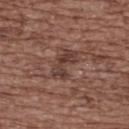notes — imaged on a skin check; not biopsied
size — ~4 mm (longest diameter)
TBP lesion metrics — a footprint of about 6 mm² and two-axis asymmetry of about 0.45; a border-irregularity index near 6/10 and a peripheral color-asymmetry measure near 1
lighting — white-light illumination
patient — female, about 75 years old
imaging modality — ~15 mm tile from a whole-body skin photo
location — the upper back